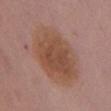{"biopsy_status": "not biopsied; imaged during a skin examination", "automated_metrics": {"area_mm2_approx": 38.0, "eccentricity": 0.75, "shape_asymmetry": 0.1, "nevus_likeness_0_100": 95, "lesion_detection_confidence_0_100": 100}, "lighting": "white-light", "image": {"source": "total-body photography crop", "field_of_view_mm": 15}, "patient": {"sex": "female", "age_approx": 50}, "lesion_size": {"long_diameter_mm_approx": 8.5}, "site": "chest"}